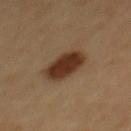workup: no biopsy performed (imaged during a skin exam)
acquisition: total-body-photography crop, ~15 mm field of view
location: the back
subject: male, aged around 50
size: ≈4.5 mm
lighting: cross-polarized
image-analysis metrics: a footprint of about 11 mm² and a shape-asymmetry score of about 0.15 (0 = symmetric); an average lesion color of about L≈33 a*≈19 b*≈29 (CIELAB) and roughly 14 lightness units darker than nearby skin; a classifier nevus-likeness of about 100/100 and lesion-presence confidence of about 100/100